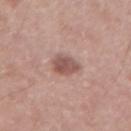The lesion was tiled from a total-body skin photograph and was not biopsied. This image is a 15 mm lesion crop taken from a total-body photograph. The tile uses white-light illumination. Located on the left forearm. A male subject, aged 43 to 47.